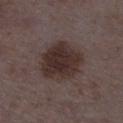A female subject, in their mid- to late 30s.
Imaged with white-light lighting.
The lesion's longest dimension is about 6 mm.
Cropped from a whole-body photographic skin survey; the tile spans about 15 mm.
The lesion is on the left lower leg.